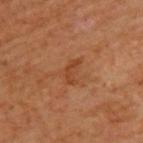  biopsy_status: not biopsied; imaged during a skin examination
  site: upper back
  lesion_size:
    long_diameter_mm_approx: 3.0
  image:
    source: total-body photography crop
    field_of_view_mm: 15
  patient:
    sex: male
    age_approx: 65
  lighting: cross-polarized